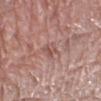  biopsy_status: not biopsied; imaged during a skin examination